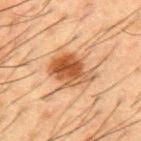<lesion>
<biopsy_status>not biopsied; imaged during a skin examination</biopsy_status>
<automated_metrics>
  <cielab_L>44</cielab_L>
  <cielab_a>20</cielab_a>
  <cielab_b>32</cielab_b>
  <vs_skin_darker_L>11.0</vs_skin_darker_L>
  <vs_skin_contrast_norm>9.0</vs_skin_contrast_norm>
  <peripheral_color_asymmetry>2.0</peripheral_color_asymmetry>
</automated_metrics>
<patient>
  <sex>male</sex>
  <age_approx>50</age_approx>
</patient>
<lesion_size>
  <long_diameter_mm_approx>5.5</long_diameter_mm_approx>
</lesion_size>
<site>mid back</site>
<lighting>cross-polarized</lighting>
<image>
  <source>total-body photography crop</source>
  <field_of_view_mm>15</field_of_view_mm>
</image>
</lesion>